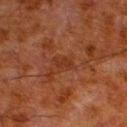notes: total-body-photography surveillance lesion; no biopsy | tile lighting: cross-polarized | location: the leg | size: about 2.5 mm | image: total-body-photography crop, ~15 mm field of view | patient: male, approximately 80 years of age.